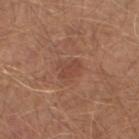Impression:
No biopsy was performed on this lesion — it was imaged during a full skin examination and was not determined to be concerning.
Background:
The recorded lesion diameter is about 3 mm. This is a white-light tile. Cropped from a whole-body photographic skin survey; the tile spans about 15 mm. On the left lower leg. A male patient, roughly 65 years of age.